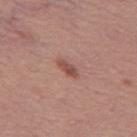Assessment: Captured during whole-body skin photography for melanoma surveillance; the lesion was not biopsied. Context: A 15 mm crop from a total-body photograph taken for skin-cancer surveillance. A female patient roughly 60 years of age. About 3 mm across. Located on the left thigh. The tile uses white-light illumination.